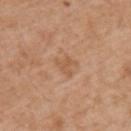This lesion was catalogued during total-body skin photography and was not selected for biopsy. Longest diameter approximately 2.5 mm. From the upper back. A region of skin cropped from a whole-body photographic capture, roughly 15 mm wide. The patient is a male in their mid-50s.